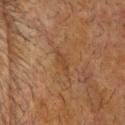Recorded during total-body skin imaging; not selected for excision or biopsy.
Automated image analysis of the tile measured a footprint of about 4 mm², an eccentricity of roughly 0.9, and two-axis asymmetry of about 0.35. It also reported a mean CIELAB color near L≈36 a*≈17 b*≈28, a lesion–skin lightness drop of about 5, and a normalized border contrast of about 5.
Cropped from a total-body skin-imaging series; the visible field is about 15 mm.
About 3.5 mm across.
A male patient about 75 years old.
On the head or neck.
The tile uses cross-polarized illumination.